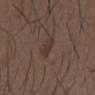  biopsy_status: not biopsied; imaged during a skin examination
  lighting: white-light
  site: mid back
  patient:
    sex: male
    age_approx: 50
  automated_metrics:
    area_mm2_approx: 3.0
    shape_asymmetry: 0.35
    vs_skin_darker_L: 7.0
    vs_skin_contrast_norm: 7.0
  image:
    source: total-body photography crop
    field_of_view_mm: 15
  lesion_size:
    long_diameter_mm_approx: 3.0